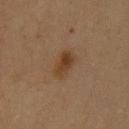| field | value |
|---|---|
| biopsy status | no biopsy performed (imaged during a skin exam) |